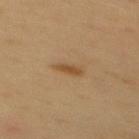workup — total-body-photography surveillance lesion; no biopsy | size — about 2.5 mm | illumination — cross-polarized | image — ~15 mm crop, total-body skin-cancer survey | body site — the back | subject — female, aged 48–52.